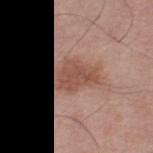biopsy status=catalogued during a skin exam; not biopsied | lighting=white-light illumination | image source=~15 mm tile from a whole-body skin photo | image-analysis metrics=a lesion area of about 13 mm², a shape eccentricity near 0.7, and a symmetry-axis asymmetry near 0.35; a nevus-likeness score of about 30/100 | patient=male, in their 70s | location=the right thigh.